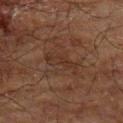Clinical impression: Part of a total-body skin-imaging series; this lesion was reviewed on a skin check and was not flagged for biopsy. Background: The tile uses cross-polarized illumination. A region of skin cropped from a whole-body photographic capture, roughly 15 mm wide. Approximately 4 mm at its widest. From the left upper arm. A male patient roughly 70 years of age.